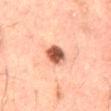Q: Was a biopsy performed?
A: no biopsy performed (imaged during a skin exam)
Q: Lesion size?
A: ≈3 mm
Q: How was this image acquired?
A: 15 mm crop, total-body photography
Q: What are the patient's age and sex?
A: male, aged approximately 50
Q: How was the tile lit?
A: cross-polarized illumination
Q: Lesion location?
A: the mid back